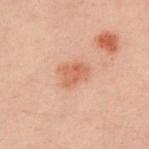A lesion tile, about 15 mm wide, cut from a 3D total-body photograph.
The lesion is on the right upper arm.
A male subject about 40 years old.
About 3 mm across.